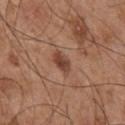<case>
<biopsy_status>not biopsied; imaged during a skin examination</biopsy_status>
<patient>
  <sex>male</sex>
  <age_approx>55</age_approx>
</patient>
<site>front of the torso</site>
<image>
  <source>total-body photography crop</source>
  <field_of_view_mm>15</field_of_view_mm>
</image>
<lighting>white-light</lighting>
<lesion_size>
  <long_diameter_mm_approx>3.0</long_diameter_mm_approx>
</lesion_size>
</case>